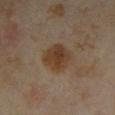follow-up — imaged on a skin check; not biopsied
subject — female, approximately 35 years of age
site — the left forearm
image source — ~15 mm crop, total-body skin-cancer survey
lesion diameter — ~3.5 mm (longest diameter)